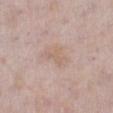{
  "site": "left lower leg",
  "image": {
    "source": "total-body photography crop",
    "field_of_view_mm": 15
  },
  "lighting": "white-light",
  "patient": {
    "sex": "female",
    "age_approx": 30
  },
  "lesion_size": {
    "long_diameter_mm_approx": 3.0
  }
}